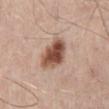Part of a total-body skin-imaging series; this lesion was reviewed on a skin check and was not flagged for biopsy. A close-up tile cropped from a whole-body skin photograph, about 15 mm across. Captured under white-light illumination. The lesion's longest dimension is about 4 mm. The lesion is on the mid back. Automated tile analysis of the lesion measured a mean CIELAB color near L≈51 a*≈21 b*≈28, roughly 17 lightness units darker than nearby skin, and a normalized lesion–skin contrast near 11. The analysis additionally found a within-lesion color-variation index near 7/10 and peripheral color asymmetry of about 2. It also reported a nevus-likeness score of about 100/100 and lesion-presence confidence of about 100/100. The patient is a male aged approximately 40.